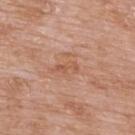Clinical impression:
No biopsy was performed on this lesion — it was imaged during a full skin examination and was not determined to be concerning.
Context:
The patient is a male in their 60s. Imaged with white-light lighting. Located on the upper back. Automated image analysis of the tile measured an automated nevus-likeness rating near 0 out of 100 and lesion-presence confidence of about 100/100. This image is a 15 mm lesion crop taken from a total-body photograph. The recorded lesion diameter is about 3 mm.